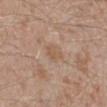Q: Was a biopsy performed?
A: no biopsy performed (imaged during a skin exam)
Q: Who is the patient?
A: male, approximately 45 years of age
Q: How large is the lesion?
A: about 2.5 mm
Q: Automated lesion metrics?
A: about 6 CIELAB-L* units darker than the surrounding skin; a border-irregularity rating of about 2/10, internal color variation of about 1.5 on a 0–10 scale, and a peripheral color-asymmetry measure near 0.5; an automated nevus-likeness rating near 0 out of 100
Q: Lesion location?
A: the right lower leg
Q: What is the imaging modality?
A: ~15 mm crop, total-body skin-cancer survey
Q: How was the tile lit?
A: white-light